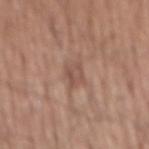The lesion was photographed on a routine skin check and not biopsied; there is no pathology result. On the mid back. A 15 mm close-up tile from a total-body photography series done for melanoma screening. The subject is a male in their mid- to late 60s.